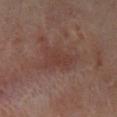• location — the right lower leg
• lesion diameter — ~4.5 mm (longest diameter)
• patient — female, roughly 50 years of age
• imaging modality — total-body-photography crop, ~15 mm field of view